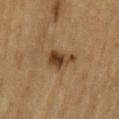notes = total-body-photography surveillance lesion; no biopsy
anatomic site = the left upper arm
image source = ~15 mm tile from a whole-body skin photo
subject = male, in their mid-80s or older